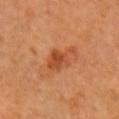Captured during whole-body skin photography for melanoma surveillance; the lesion was not biopsied. A subject aged 68–72. About 4.5 mm across. A region of skin cropped from a whole-body photographic capture, roughly 15 mm wide. An algorithmic analysis of the crop reported an area of roughly 7.5 mm², an outline eccentricity of about 0.8 (0 = round, 1 = elongated), and a shape-asymmetry score of about 0.4 (0 = symmetric). And it measured an average lesion color of about L≈49 a*≈29 b*≈39 (CIELAB), roughly 9 lightness units darker than nearby skin, and a normalized lesion–skin contrast near 7. The analysis additionally found border irregularity of about 4.5 on a 0–10 scale, a color-variation rating of about 3.5/10, and radial color variation of about 1. It also reported a classifier nevus-likeness of about 65/100 and a detector confidence of about 100 out of 100 that the crop contains a lesion. The lesion is on the right upper arm.